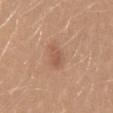workup: imaged on a skin check; not biopsied
subject: female, approximately 30 years of age
size: ~2.5 mm (longest diameter)
TBP lesion metrics: a normalized lesion–skin contrast near 5.5; an automated nevus-likeness rating near 45 out of 100 and a detector confidence of about 100 out of 100 that the crop contains a lesion
image source: ~15 mm tile from a whole-body skin photo
lighting: white-light
location: the back